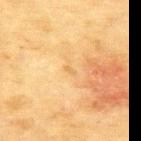This lesion was catalogued during total-body skin photography and was not selected for biopsy. The subject is a female about 80 years old. The total-body-photography lesion software estimated a lesion color around L≈61 a*≈16 b*≈42 in CIELAB, roughly 5 lightness units darker than nearby skin, and a normalized border contrast of about 3.5. It also reported border irregularity of about 3.5 on a 0–10 scale and a color-variation rating of about 0/10. Cropped from a whole-body photographic skin survey; the tile spans about 15 mm. This is a cross-polarized tile. The lesion is on the back. The recorded lesion diameter is about 1.5 mm.